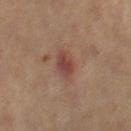  biopsy_status: not biopsied; imaged during a skin examination
  automated_metrics:
    area_mm2_approx: 6.0
    eccentricity: 0.75
    border_irregularity_0_10: 1.5
    color_variation_0_10: 2.5
    peripheral_color_asymmetry: 0.5
    nevus_likeness_0_100: 75
    lesion_detection_confidence_0_100: 100
  site: right thigh
  lesion_size:
    long_diameter_mm_approx: 3.0
  lighting: cross-polarized
  patient:
    sex: female
    age_approx: 40
  image:
    source: total-body photography crop
    field_of_view_mm: 15